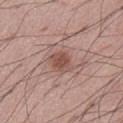Assessment: The lesion was tiled from a total-body skin photograph and was not biopsied. Context: Measured at roughly 3 mm in maximum diameter. A 15 mm crop from a total-body photograph taken for skin-cancer surveillance. Automated tile analysis of the lesion measured a shape eccentricity near 0.6 and a shape-asymmetry score of about 0.15 (0 = symmetric). It also reported roughly 10 lightness units darker than nearby skin. The software also gave a border-irregularity index near 1.5/10 and peripheral color asymmetry of about 1. The patient is a male in their mid- to late 50s. On the abdomen.